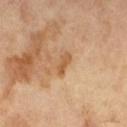Q: Was a biopsy performed?
A: catalogued during a skin exam; not biopsied
Q: How was this image acquired?
A: 15 mm crop, total-body photography
Q: What is the lesion's diameter?
A: ~3 mm (longest diameter)
Q: Illumination type?
A: cross-polarized illumination
Q: What are the patient's age and sex?
A: male, aged around 65